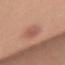Imaged during a routine full-body skin examination; the lesion was not biopsied and no histopathology is available. The recorded lesion diameter is about 4 mm. Imaged with white-light lighting. A female patient approximately 35 years of age. The lesion is located on the mid back. A 15 mm close-up tile from a total-body photography series done for melanoma screening. The total-body-photography lesion software estimated a lesion area of about 6.5 mm² and a shape-asymmetry score of about 0.2 (0 = symmetric). The software also gave an average lesion color of about L≈56 a*≈24 b*≈28 (CIELAB), a lesion–skin lightness drop of about 8, and a lesion-to-skin contrast of about 6 (normalized; higher = more distinct). It also reported a border-irregularity rating of about 2/10, a within-lesion color-variation index near 3.5/10, and radial color variation of about 1.5. The analysis additionally found an automated nevus-likeness rating near 10 out of 100.